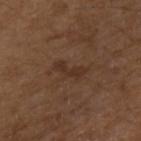The lesion was tiled from a total-body skin photograph and was not biopsied. Automated tile analysis of the lesion measured roughly 6 lightness units darker than nearby skin and a lesion-to-skin contrast of about 6 (normalized; higher = more distinct). It also reported a nevus-likeness score of about 0/100 and a lesion-detection confidence of about 100/100. A male patient roughly 75 years of age. From the right upper arm. Captured under white-light illumination. Cropped from a total-body skin-imaging series; the visible field is about 15 mm.